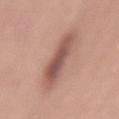biopsy_status: not biopsied; imaged during a skin examination
lesion_size:
  long_diameter_mm_approx: 6.0
site: left thigh
image:
  source: total-body photography crop
  field_of_view_mm: 15
patient:
  sex: male
  age_approx: 50
automated_metrics:
  area_mm2_approx: 16.0
  eccentricity: 0.85
  border_irregularity_0_10: 2.5
lighting: white-light